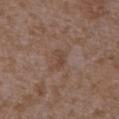subject — female, aged around 35 | location — the arm | acquisition — total-body-photography crop, ~15 mm field of view.